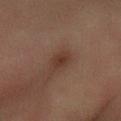Clinical impression:
Recorded during total-body skin imaging; not selected for excision or biopsy.
Context:
About 2.5 mm across. The lesion is on the right forearm. The total-body-photography lesion software estimated a lesion area of about 4 mm², an outline eccentricity of about 0.7 (0 = round, 1 = elongated), and a shape-asymmetry score of about 0.15 (0 = symmetric). It also reported an automated nevus-likeness rating near 30 out of 100 and lesion-presence confidence of about 100/100. A male subject, in their mid- to late 50s. A 15 mm crop from a total-body photograph taken for skin-cancer surveillance.